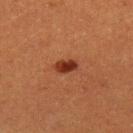Recorded during total-body skin imaging; not selected for excision or biopsy.
A roughly 15 mm field-of-view crop from a total-body skin photograph.
A male subject, about 40 years old.
The tile uses cross-polarized illumination.
On the arm.
Longest diameter approximately 3 mm.